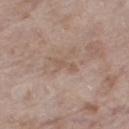{
  "biopsy_status": "not biopsied; imaged during a skin examination",
  "lesion_size": {
    "long_diameter_mm_approx": 3.0
  },
  "lighting": "white-light",
  "image": {
    "source": "total-body photography crop",
    "field_of_view_mm": 15
  },
  "patient": {
    "sex": "female",
    "age_approx": 75
  },
  "site": "right thigh"
}